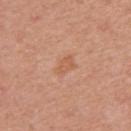  patient:
    sex: female
    age_approx: 60
  lesion_size:
    long_diameter_mm_approx: 2.5
  lighting: white-light
  site: right upper arm
  automated_metrics:
    area_mm2_approx: 3.5
    eccentricity: 0.8
    shape_asymmetry: 0.35
    cielab_L: 58
    cielab_a: 24
    cielab_b: 34
    vs_skin_darker_L: 7.0
    vs_skin_contrast_norm: 5.0
    border_irregularity_0_10: 3.5
    color_variation_0_10: 1.5
    peripheral_color_asymmetry: 0.5
    nevus_likeness_0_100: 5
    lesion_detection_confidence_0_100: 100
  image:
    source: total-body photography crop
    field_of_view_mm: 15